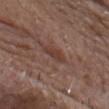Image and clinical context: The tile uses white-light illumination. Automated tile analysis of the lesion measured a nevus-likeness score of about 5/100 and a detector confidence of about 100 out of 100 that the crop contains a lesion. Longest diameter approximately 3 mm. A male subject, aged 78–82. Located on the chest. A roughly 15 mm field-of-view crop from a total-body skin photograph.